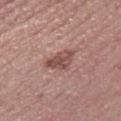Clinical impression: No biopsy was performed on this lesion — it was imaged during a full skin examination and was not determined to be concerning. Clinical summary: A close-up tile cropped from a whole-body skin photograph, about 15 mm across. A female subject, aged 48 to 52. The lesion is on the right thigh. An algorithmic analysis of the crop reported a footprint of about 7 mm², an outline eccentricity of about 0.75 (0 = round, 1 = elongated), and a symmetry-axis asymmetry near 0.4. And it measured a border-irregularity rating of about 4/10. Longest diameter approximately 3.5 mm.